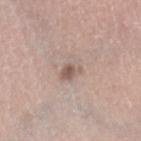Clinical impression: Part of a total-body skin-imaging series; this lesion was reviewed on a skin check and was not flagged for biopsy. Clinical summary: Approximately 3 mm at its widest. A 15 mm crop from a total-body photograph taken for skin-cancer surveillance. An algorithmic analysis of the crop reported a border-irregularity rating of about 3/10 and a peripheral color-asymmetry measure near 2. And it measured a nevus-likeness score of about 0/100 and lesion-presence confidence of about 100/100. Located on the right lower leg. Captured under white-light illumination. A female subject, about 40 years old.